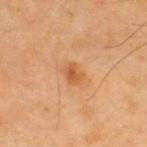Clinical impression: Imaged during a routine full-body skin examination; the lesion was not biopsied and no histopathology is available. Context: The subject is a male aged 38 to 42. This image is a 15 mm lesion crop taken from a total-body photograph. The tile uses cross-polarized illumination. The lesion is on the upper back.